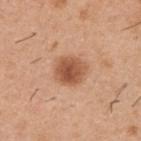- biopsy status — no biopsy performed (imaged during a skin exam)
- image — 15 mm crop, total-body photography
- patient — male, aged approximately 40
- lighting — white-light illumination
- lesion size — about 3.5 mm
- body site — the upper back